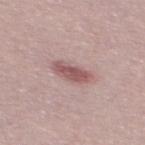The lesion was tiled from a total-body skin photograph and was not biopsied. The patient is a male aged 28 to 32. The lesion's longest dimension is about 4 mm. A lesion tile, about 15 mm wide, cut from a 3D total-body photograph.